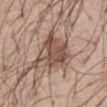| key | value |
|---|---|
| subject | male, about 55 years old |
| acquisition | 15 mm crop, total-body photography |
| diameter | about 6 mm |
| anatomic site | the chest |